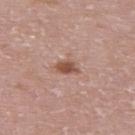workup=catalogued during a skin exam; not biopsied
illumination=white-light illumination
location=the upper back
size=about 2.5 mm
image source=15 mm crop, total-body photography
subject=female, about 30 years old